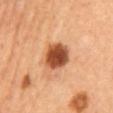This lesion was catalogued during total-body skin photography and was not selected for biopsy. Cropped from a whole-body photographic skin survey; the tile spans about 15 mm. Automated tile analysis of the lesion measured an area of roughly 10 mm² and an eccentricity of roughly 0.65. The software also gave about 19 CIELAB-L* units darker than the surrounding skin and a normalized border contrast of about 12.5. Approximately 4 mm at its widest. The patient is female. The tile uses cross-polarized illumination. The lesion is located on the abdomen.